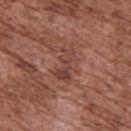This lesion was catalogued during total-body skin photography and was not selected for biopsy. A 15 mm close-up tile from a total-body photography series done for melanoma screening. The lesion is located on the back. The subject is a male aged approximately 75. This is a white-light tile.